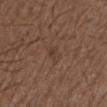Impression:
No biopsy was performed on this lesion — it was imaged during a full skin examination and was not determined to be concerning.
Image and clinical context:
The recorded lesion diameter is about 2.5 mm. The tile uses white-light illumination. Automated image analysis of the tile measured a footprint of about 3 mm², an eccentricity of roughly 0.85, and a symmetry-axis asymmetry near 0.25. And it measured a nevus-likeness score of about 0/100 and a lesion-detection confidence of about 100/100. A male subject approximately 50 years of age. From the left upper arm. A roughly 15 mm field-of-view crop from a total-body skin photograph.